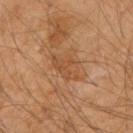Notes:
– location: the right upper arm
– tile lighting: cross-polarized
– image-analysis metrics: a shape eccentricity near 0.75 and a symmetry-axis asymmetry near 0.35; an average lesion color of about L≈50 a*≈22 b*≈36 (CIELAB), roughly 7 lightness units darker than nearby skin, and a lesion-to-skin contrast of about 5.5 (normalized; higher = more distinct); a border-irregularity rating of about 4/10 and radial color variation of about 1
– size: ≈4 mm
– subject: male, aged around 50
– image: ~15 mm crop, total-body skin-cancer survey